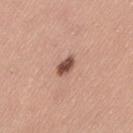No biopsy was performed on this lesion — it was imaged during a full skin examination and was not determined to be concerning. The subject is a female in their mid-40s. A region of skin cropped from a whole-body photographic capture, roughly 15 mm wide. Captured under white-light illumination. Automated image analysis of the tile measured a lesion color around L≈50 a*≈23 b*≈27 in CIELAB, a lesion–skin lightness drop of about 17, and a normalized lesion–skin contrast near 11. And it measured a border-irregularity rating of about 2.5/10, a color-variation rating of about 2.5/10, and a peripheral color-asymmetry measure near 0.5. From the left thigh.